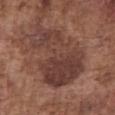A close-up tile cropped from a whole-body skin photograph, about 15 mm across.
Longest diameter approximately 9 mm.
The lesion is located on the abdomen.
The tile uses white-light illumination.
A male subject aged around 75.